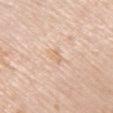Q: Is there a histopathology result?
A: no biopsy performed (imaged during a skin exam)
Q: Who is the patient?
A: female, about 65 years old
Q: Where on the body is the lesion?
A: the right upper arm
Q: Lesion size?
A: ~2.5 mm (longest diameter)
Q: What is the imaging modality?
A: total-body-photography crop, ~15 mm field of view
Q: How was the tile lit?
A: white-light illumination
Q: Automated lesion metrics?
A: an automated nevus-likeness rating near 0 out of 100 and lesion-presence confidence of about 100/100